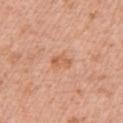Q: Is there a histopathology result?
A: no biopsy performed (imaged during a skin exam)
Q: How was this image acquired?
A: ~15 mm tile from a whole-body skin photo
Q: Lesion location?
A: the chest
Q: How large is the lesion?
A: ~3 mm (longest diameter)
Q: Illumination type?
A: white-light illumination
Q: What are the patient's age and sex?
A: female, aged around 40